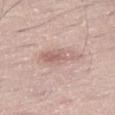<tbp_lesion>
  <biopsy_status>not biopsied; imaged during a skin examination</biopsy_status>
  <automated_metrics>
    <area_mm2_approx>9.0</area_mm2_approx>
    <eccentricity>0.9</eccentricity>
    <shape_asymmetry>0.2</shape_asymmetry>
    <vs_skin_darker_L>9.0</vs_skin_darker_L>
    <vs_skin_contrast_norm>5.5</vs_skin_contrast_norm>
    <nevus_likeness_0_100>0</nevus_likeness_0_100>
    <lesion_detection_confidence_0_100>100</lesion_detection_confidence_0_100>
  </automated_metrics>
  <image>
    <source>total-body photography crop</source>
    <field_of_view_mm>15</field_of_view_mm>
  </image>
  <site>leg</site>
  <lesion_size>
    <long_diameter_mm_approx>5.0</long_diameter_mm_approx>
  </lesion_size>
  <patient>
    <sex>male</sex>
    <age_approx>55</age_approx>
  </patient>
  <lighting>white-light</lighting>
</tbp_lesion>